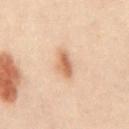Acquisition and patient details:
The patient is a male aged around 50. On the abdomen. A 15 mm crop from a total-body photograph taken for skin-cancer surveillance.